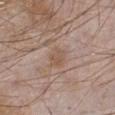This lesion was catalogued during total-body skin photography and was not selected for biopsy. This image is a 15 mm lesion crop taken from a total-body photograph. Imaged with white-light lighting. A male patient, aged 63–67. From the left lower leg.